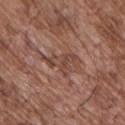The lesion is on the chest.
Measured at roughly 4.5 mm in maximum diameter.
This image is a 15 mm lesion crop taken from a total-body photograph.
The subject is a male approximately 70 years of age.
This is a white-light tile.